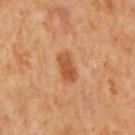<record>
  <biopsy_status>not biopsied; imaged during a skin examination</biopsy_status>
  <image>
    <source>total-body photography crop</source>
    <field_of_view_mm>15</field_of_view_mm>
  </image>
  <site>mid back</site>
  <lighting>cross-polarized</lighting>
  <patient>
    <sex>male</sex>
    <age_approx>65</age_approx>
  </patient>
  <lesion_size>
    <long_diameter_mm_approx>3.5</long_diameter_mm_approx>
  </lesion_size>
</record>